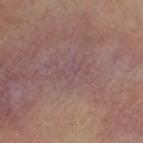| feature | finding |
|---|---|
| lesion size | ~3.5 mm (longest diameter) |
| acquisition | total-body-photography crop, ~15 mm field of view |
| subject | female |
| automated lesion analysis | an area of roughly 5 mm²; border irregularity of about 7.5 on a 0–10 scale, a color-variation rating of about 2/10, and peripheral color asymmetry of about 0.5; a classifier nevus-likeness of about 0/100 and a detector confidence of about 60 out of 100 that the crop contains a lesion |